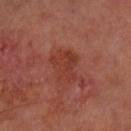biopsy status: no biopsy performed (imaged during a skin exam)
acquisition: 15 mm crop, total-body photography
anatomic site: the right forearm
size: ≈4 mm
patient: male, aged around 70